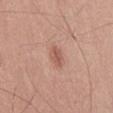illumination: white-light; site: the leg; subject: male, aged 73 to 77; lesion size: ~3 mm (longest diameter); image-analysis metrics: a lesion color around L≈56 a*≈24 b*≈28 in CIELAB, roughly 9 lightness units darker than nearby skin, and a normalized lesion–skin contrast near 6.5; acquisition: total-body-photography crop, ~15 mm field of view.